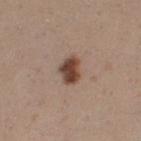biopsy status=no biopsy performed (imaged during a skin exam)